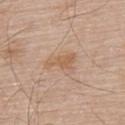Case summary:
* biopsy status: no biopsy performed (imaged during a skin exam)
* body site: the upper back
* automated metrics: a lesion color around L≈59 a*≈19 b*≈33 in CIELAB, roughly 7 lightness units darker than nearby skin, and a normalized lesion–skin contrast near 6.5; a color-variation rating of about 1.5/10 and peripheral color asymmetry of about 0.5; a nevus-likeness score of about 5/100 and a detector confidence of about 100 out of 100 that the crop contains a lesion
* illumination: white-light
* image: 15 mm crop, total-body photography
* diameter: ~3.5 mm (longest diameter)
* subject: male, in their 80s